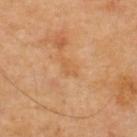Q: Was this lesion biopsied?
A: total-body-photography surveillance lesion; no biopsy
Q: What kind of image is this?
A: ~15 mm crop, total-body skin-cancer survey
Q: Who is the patient?
A: male, about 70 years old
Q: What is the lesion's diameter?
A: ≈2.5 mm
Q: What is the anatomic site?
A: the upper back